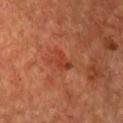| key | value |
|---|---|
| follow-up | no biopsy performed (imaged during a skin exam) |
| lesion diameter | ≈3 mm |
| image source | ~15 mm tile from a whole-body skin photo |
| subject | male, aged around 50 |
| automated lesion analysis | a mean CIELAB color near L≈42 a*≈31 b*≈36, a lesion–skin lightness drop of about 7, and a normalized border contrast of about 6; a border-irregularity rating of about 5/10 and peripheral color asymmetry of about 0.5 |
| anatomic site | the chest |
| tile lighting | cross-polarized |